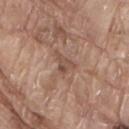<lesion>
<biopsy_status>not biopsied; imaged during a skin examination</biopsy_status>
<image>
  <source>total-body photography crop</source>
  <field_of_view_mm>15</field_of_view_mm>
</image>
<lesion_size>
  <long_diameter_mm_approx>2.5</long_diameter_mm_approx>
</lesion_size>
<patient>
  <sex>male</sex>
  <age_approx>80</age_approx>
</patient>
<site>lower back</site>
</lesion>